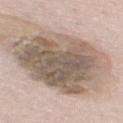| feature | finding |
|---|---|
| workup | total-body-photography surveillance lesion; no biopsy |
| tile lighting | white-light |
| diameter | ~10 mm (longest diameter) |
| imaging modality | ~15 mm tile from a whole-body skin photo |
| patient | male, aged approximately 55 |
| site | the mid back |